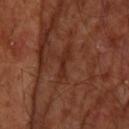| field | value |
|---|---|
| follow-up | catalogued during a skin exam; not biopsied |
| size | ~3 mm (longest diameter) |
| illumination | cross-polarized |
| subject | male, approximately 55 years of age |
| site | the upper back |
| imaging modality | ~15 mm tile from a whole-body skin photo |
| automated lesion analysis | an area of roughly 2 mm², an outline eccentricity of about 0.95 (0 = round, 1 = elongated), and a shape-asymmetry score of about 0.6 (0 = symmetric); an automated nevus-likeness rating near 0 out of 100 and a lesion-detection confidence of about 55/100 |